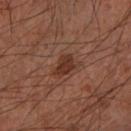<case>
<biopsy_status>not biopsied; imaged during a skin examination</biopsy_status>
<patient>
  <sex>male</sex>
  <age_approx>65</age_approx>
</patient>
<site>left forearm</site>
<image>
  <source>total-body photography crop</source>
  <field_of_view_mm>15</field_of_view_mm>
</image>
<lesion_size>
  <long_diameter_mm_approx>3.0</long_diameter_mm_approx>
</lesion_size>
</case>